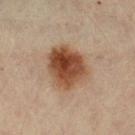Q: Was a biopsy performed?
A: total-body-photography surveillance lesion; no biopsy
Q: What lighting was used for the tile?
A: cross-polarized illumination
Q: Where on the body is the lesion?
A: the right thigh
Q: Who is the patient?
A: female, in their 60s
Q: What kind of image is this?
A: total-body-photography crop, ~15 mm field of view
Q: How large is the lesion?
A: ~5.5 mm (longest diameter)
Q: What did automated image analysis measure?
A: a footprint of about 20 mm² and an outline eccentricity of about 0.6 (0 = round, 1 = elongated); a border-irregularity rating of about 2/10 and a within-lesion color-variation index near 9/10